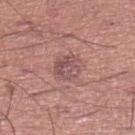Recorded during total-body skin imaging; not selected for excision or biopsy. A male subject, in their mid-40s. From the right lower leg. This is a white-light tile. The lesion-visualizer software estimated an area of roughly 7.5 mm², an outline eccentricity of about 0.3 (0 = round, 1 = elongated), and a symmetry-axis asymmetry near 0.2. The software also gave a lesion–skin lightness drop of about 8. It also reported a border-irregularity index near 2/10 and radial color variation of about 2. It also reported a classifier nevus-likeness of about 0/100 and a lesion-detection confidence of about 100/100. The recorded lesion diameter is about 3 mm. A 15 mm crop from a total-body photograph taken for skin-cancer surveillance.